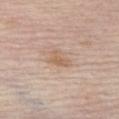Findings:
– acquisition — 15 mm crop, total-body photography
– body site — the left thigh
– subject — male, aged approximately 85 or older